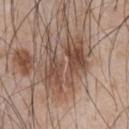No biopsy was performed on this lesion — it was imaged during a full skin examination and was not determined to be concerning. The lesion is located on the front of the torso. Imaged with white-light lighting. A lesion tile, about 15 mm wide, cut from a 3D total-body photograph. The patient is a male in their mid- to late 40s. The recorded lesion diameter is about 7.5 mm.